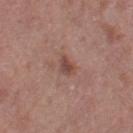workup=catalogued during a skin exam; not biopsied
imaging modality=15 mm crop, total-body photography
subject=female, in their 40s
anatomic site=the left thigh